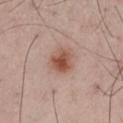Recorded during total-body skin imaging; not selected for excision or biopsy. Located on the leg. Cropped from a whole-body photographic skin survey; the tile spans about 15 mm. The patient is a male aged around 75. The lesion-visualizer software estimated an area of roughly 7.5 mm² and an eccentricity of roughly 0.6. It also reported an average lesion color of about L≈53 a*≈22 b*≈28 (CIELAB), a lesion–skin lightness drop of about 12, and a normalized lesion–skin contrast near 9. The analysis additionally found a border-irregularity index near 2/10, a within-lesion color-variation index near 5/10, and radial color variation of about 1.5. It also reported a nevus-likeness score of about 95/100 and lesion-presence confidence of about 100/100. Longest diameter approximately 3.5 mm.